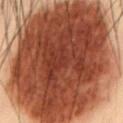Notes:
- workup — total-body-photography surveillance lesion; no biopsy
- diameter — ≈16 mm
- anatomic site — the abdomen
- imaging modality — ~15 mm tile from a whole-body skin photo
- illumination — cross-polarized illumination
- subject — male, in their mid-50s